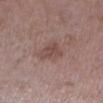<lesion>
<biopsy_status>not biopsied; imaged during a skin examination</biopsy_status>
<site>left lower leg</site>
<lesion_size>
  <long_diameter_mm_approx>3.0</long_diameter_mm_approx>
</lesion_size>
<patient>
  <sex>male</sex>
  <age_approx>70</age_approx>
</patient>
<image>
  <source>total-body photography crop</source>
  <field_of_view_mm>15</field_of_view_mm>
</image>
<automated_metrics>
  <area_mm2_approx>6.5</area_mm2_approx>
  <eccentricity>0.55</eccentricity>
  <cielab_L>47</cielab_L>
  <cielab_a>19</cielab_a>
  <cielab_b>21</cielab_b>
  <vs_skin_darker_L>8.0</vs_skin_darker_L>
  <vs_skin_contrast_norm>6.0</vs_skin_contrast_norm>
  <border_irregularity_0_10>4.0</border_irregularity_0_10>
  <peripheral_color_asymmetry>1.0</peripheral_color_asymmetry>
</automated_metrics>
<lighting>white-light</lighting>
</lesion>